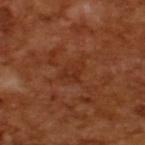<record>
<image>
  <source>total-body photography crop</source>
  <field_of_view_mm>15</field_of_view_mm>
</image>
<patient>
  <sex>male</sex>
  <age_approx>65</age_approx>
</patient>
<automated_metrics>
  <nevus_likeness_0_100>0</nevus_likeness_0_100>
</automated_metrics>
<lesion_size>
  <long_diameter_mm_approx>3.5</long_diameter_mm_approx>
</lesion_size>
<lighting>cross-polarized</lighting>
</record>